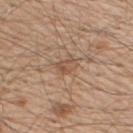workup: imaged on a skin check; not biopsied
acquisition: total-body-photography crop, ~15 mm field of view
subject: male, roughly 55 years of age
body site: the upper back
diameter: ≈2.5 mm
illumination: white-light
image-analysis metrics: a footprint of about 3.5 mm², an outline eccentricity of about 0.8 (0 = round, 1 = elongated), and a symmetry-axis asymmetry near 0.35; a within-lesion color-variation index near 4.5/10 and radial color variation of about 2; lesion-presence confidence of about 100/100